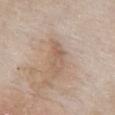Assessment: Part of a total-body skin-imaging series; this lesion was reviewed on a skin check and was not flagged for biopsy. Background: Located on the chest. Imaged with white-light lighting. A 15 mm close-up tile from a total-body photography series done for melanoma screening. A female patient, aged approximately 75. About 4 mm across.